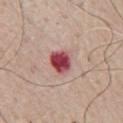| field | value |
|---|---|
| image | 15 mm crop, total-body photography |
| subject | male, about 70 years old |
| anatomic site | the chest |
| image-analysis metrics | a shape eccentricity near 0.5; a mean CIELAB color near L≈48 a*≈33 b*≈21, about 19 CIELAB-L* units darker than the surrounding skin, and a lesion-to-skin contrast of about 13 (normalized; higher = more distinct); a within-lesion color-variation index near 6/10; a classifier nevus-likeness of about 0/100 |